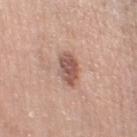{"biopsy_status": "not biopsied; imaged during a skin examination", "patient": {"sex": "female", "age_approx": 40}, "image": {"source": "total-body photography crop", "field_of_view_mm": 15}, "site": "left thigh", "automated_metrics": {"area_mm2_approx": 7.0, "eccentricity": 0.85, "shape_asymmetry": 0.15, "vs_skin_darker_L": 13.0, "vs_skin_contrast_norm": 8.5, "border_irregularity_0_10": 1.5, "color_variation_0_10": 3.5, "peripheral_color_asymmetry": 1.0, "nevus_likeness_0_100": 80, "lesion_detection_confidence_0_100": 100}}